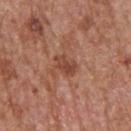{
  "biopsy_status": "not biopsied; imaged during a skin examination"
}